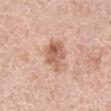This lesion was catalogued during total-body skin photography and was not selected for biopsy. The recorded lesion diameter is about 3.5 mm. Imaged with white-light lighting. Located on the right lower leg. A close-up tile cropped from a whole-body skin photograph, about 15 mm across. The lesion-visualizer software estimated a footprint of about 9 mm². The software also gave an average lesion color of about L≈61 a*≈22 b*≈30 (CIELAB), roughly 12 lightness units darker than nearby skin, and a normalized lesion–skin contrast near 7.5. The analysis additionally found an automated nevus-likeness rating near 45 out of 100. A female subject, aged 63 to 67.